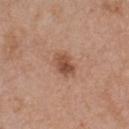Imaged during a routine full-body skin examination; the lesion was not biopsied and no histopathology is available.
Automated image analysis of the tile measured a lesion area of about 5.5 mm², a shape eccentricity near 0.75, and two-axis asymmetry of about 0.25.
A female subject, aged 38 to 42.
About 3 mm across.
This is a white-light tile.
On the chest.
A 15 mm close-up tile from a total-body photography series done for melanoma screening.